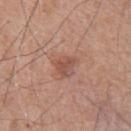{"biopsy_status": "not biopsied; imaged during a skin examination", "image": {"source": "total-body photography crop", "field_of_view_mm": 15}, "site": "upper back", "lesion_size": {"long_diameter_mm_approx": 2.5}, "patient": {"sex": "male", "age_approx": 65}}